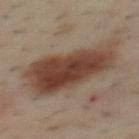biopsy_status: not biopsied; imaged during a skin examination
patient:
  sex: male
  age_approx: 40
lesion_size:
  long_diameter_mm_approx: 9.0
site: mid back
lighting: cross-polarized
image:
  source: total-body photography crop
  field_of_view_mm: 15